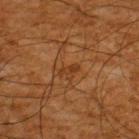Background:
Cropped from a whole-body photographic skin survey; the tile spans about 15 mm. The patient is a male in their mid- to late 60s. Located on the upper back. Measured at roughly 2.5 mm in maximum diameter. The tile uses cross-polarized illumination.